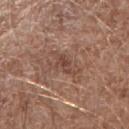Findings:
– biopsy status: imaged on a skin check; not biopsied
– patient: male, aged approximately 70
– diameter: ≈3 mm
– location: the right forearm
– image source: ~15 mm crop, total-body skin-cancer survey